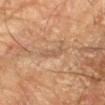notes = imaged on a skin check; not biopsied
location = the left forearm
imaging modality = ~15 mm crop, total-body skin-cancer survey
automated lesion analysis = a lesion area of about 3.5 mm², a shape eccentricity near 0.9, and two-axis asymmetry of about 0.3
subject = male, aged 63–67
lesion diameter = about 3 mm
lighting = cross-polarized